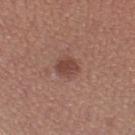Findings:
* notes: no biopsy performed (imaged during a skin exam)
* automated metrics: a lesion–skin lightness drop of about 10 and a normalized lesion–skin contrast near 7.5
* acquisition: ~15 mm tile from a whole-body skin photo
* anatomic site: the right lower leg
* lesion diameter: about 2.5 mm
* subject: female, aged around 35
* tile lighting: white-light illumination